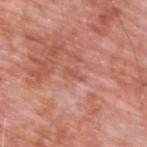Clinical impression:
Recorded during total-body skin imaging; not selected for excision or biopsy.
Image and clinical context:
A 15 mm close-up extracted from a 3D total-body photography capture. Captured under white-light illumination. Located on the upper back. A male subject, approximately 60 years of age.